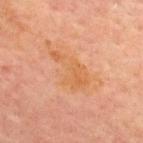{
  "biopsy_status": "not biopsied; imaged during a skin examination",
  "site": "front of the torso",
  "lighting": "cross-polarized",
  "patient": {
    "sex": "male",
    "age_approx": 70
  },
  "lesion_size": {
    "long_diameter_mm_approx": 5.5
  },
  "image": {
    "source": "total-body photography crop",
    "field_of_view_mm": 15
  }
}